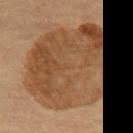The lesion was tiled from a total-body skin photograph and was not biopsied. Captured under cross-polarized illumination. Automated image analysis of the tile measured a lesion area of about 80 mm², an outline eccentricity of about 0.65 (0 = round, 1 = elongated), and a symmetry-axis asymmetry near 0.15. The software also gave a classifier nevus-likeness of about 85/100 and a lesion-detection confidence of about 65/100. The lesion is on the abdomen. A 15 mm crop from a total-body photograph taken for skin-cancer surveillance. A male patient aged 58 to 62. Longest diameter approximately 11.5 mm.